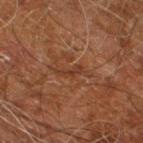Part of a total-body skin-imaging series; this lesion was reviewed on a skin check and was not flagged for biopsy. This image is a 15 mm lesion crop taken from a total-body photograph. On the right leg. A male patient about 60 years old.